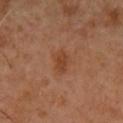biopsy status = total-body-photography surveillance lesion; no biopsy | automated lesion analysis = an average lesion color of about L≈42 a*≈23 b*≈33 (CIELAB), roughly 7 lightness units darker than nearby skin, and a normalized lesion–skin contrast near 6.5; a classifier nevus-likeness of about 35/100 | anatomic site = the chest | image source = ~15 mm crop, total-body skin-cancer survey | subject = male, about 50 years old.